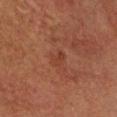Part of a total-body skin-imaging series; this lesion was reviewed on a skin check and was not flagged for biopsy. A 15 mm close-up tile from a total-body photography series done for melanoma screening. On the head or neck. The recorded lesion diameter is about 2.5 mm. Imaged with cross-polarized lighting. A male patient, approximately 60 years of age.